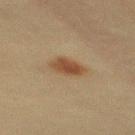A 15 mm close-up extracted from a 3D total-body photography capture.
Approximately 3.5 mm at its widest.
Captured under cross-polarized illumination.
A female subject, aged 38 to 42.
The lesion is on the mid back.
The total-body-photography lesion software estimated an area of roughly 6 mm², an eccentricity of roughly 0.75, and two-axis asymmetry of about 0.3. It also reported a border-irregularity index near 2.5/10. The analysis additionally found a classifier nevus-likeness of about 100/100 and a detector confidence of about 100 out of 100 that the crop contains a lesion.